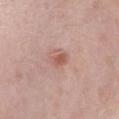imaging modality: ~15 mm tile from a whole-body skin photo | TBP lesion metrics: a color-variation rating of about 1.5/10; a classifier nevus-likeness of about 30/100 and lesion-presence confidence of about 100/100 | patient: female, in their mid- to late 40s | body site: the right forearm | lesion diameter: ~2.5 mm (longest diameter) | lighting: white-light.